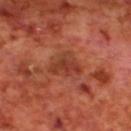Case summary:
– workup: catalogued during a skin exam; not biopsied
– image-analysis metrics: an area of roughly 8 mm²; about 8 CIELAB-L* units darker than the surrounding skin and a normalized border contrast of about 6.5; a lesion-detection confidence of about 100/100
– lighting: cross-polarized illumination
– body site: the back
– imaging modality: ~15 mm tile from a whole-body skin photo
– subject: male, aged 68 to 72